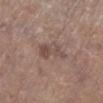This lesion was catalogued during total-body skin photography and was not selected for biopsy. A 15 mm close-up extracted from a 3D total-body photography capture. Imaged with white-light lighting. The subject is a male roughly 65 years of age. The lesion is located on the leg.